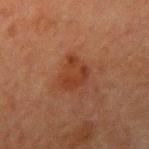follow-up: imaged on a skin check; not biopsied
subject: male, approximately 65 years of age
body site: the left upper arm
image source: ~15 mm crop, total-body skin-cancer survey